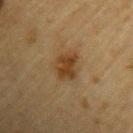{
  "image": {
    "source": "total-body photography crop",
    "field_of_view_mm": 15
  },
  "site": "left upper arm",
  "patient": {
    "sex": "male",
    "age_approx": "85+"
  }
}